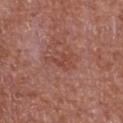Impression: The lesion was tiled from a total-body skin photograph and was not biopsied. Background: From the chest. A region of skin cropped from a whole-body photographic capture, roughly 15 mm wide. A male subject, aged around 65.